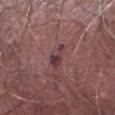| key | value |
|---|---|
| imaging modality | ~15 mm tile from a whole-body skin photo |
| location | the left thigh |
| patient | male, aged 78 to 82 |